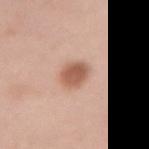follow-up=imaged on a skin check; not biopsied
TBP lesion metrics=a lesion color around L≈58 a*≈22 b*≈30 in CIELAB, roughly 13 lightness units darker than nearby skin, and a normalized lesion–skin contrast near 8.5; a border-irregularity rating of about 2/10, a within-lesion color-variation index near 3/10, and a peripheral color-asymmetry measure near 1; a nevus-likeness score of about 100/100
image=15 mm crop, total-body photography
size=~3.5 mm (longest diameter)
patient=female, roughly 50 years of age
illumination=white-light illumination
location=the right forearm